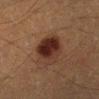The lesion was photographed on a routine skin check and not biopsied; there is no pathology result. The lesion is located on the leg. Automated tile analysis of the lesion measured an average lesion color of about L≈24 a*≈17 b*≈21 (CIELAB), a lesion–skin lightness drop of about 11, and a normalized lesion–skin contrast near 11.5. It also reported a border-irregularity index near 1.5/10 and radial color variation of about 2.5. It also reported an automated nevus-likeness rating near 100 out of 100 and lesion-presence confidence of about 100/100. The patient is a male approximately 40 years of age. The tile uses cross-polarized illumination. A 15 mm crop from a total-body photograph taken for skin-cancer surveillance.